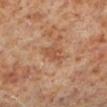imaging modality = 15 mm crop, total-body photography
anatomic site = the left lower leg
subject = male, in their 60s